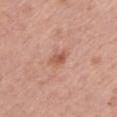notes: catalogued during a skin exam; not biopsied
lighting: white-light illumination
automated metrics: an area of roughly 3 mm², an outline eccentricity of about 0.8 (0 = round, 1 = elongated), and two-axis asymmetry of about 0.25; a mean CIELAB color near L≈56 a*≈24 b*≈31, roughly 10 lightness units darker than nearby skin, and a normalized border contrast of about 7
body site: the mid back
patient: female, aged around 65
acquisition: ~15 mm crop, total-body skin-cancer survey